A female patient roughly 45 years of age · captured under white-light illumination · the lesion is located on the left thigh · The lesion-visualizer software estimated an average lesion color of about L≈46 a*≈17 b*≈21 (CIELAB), roughly 22 lightness units darker than nearby skin, and a normalized lesion–skin contrast near 14.5. It also reported a border-irregularity rating of about 2/10, internal color variation of about 5.5 on a 0–10 scale, and peripheral color asymmetry of about 1.5 · cropped from a whole-body photographic skin survey; the tile spans about 15 mm.
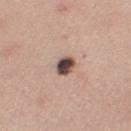{
  "diagnosis": {
    "histopathology": "dysplastic (Clark) nevus",
    "malignancy": "benign",
    "taxonomic_path": [
      "Benign",
      "Benign melanocytic proliferations",
      "Nevus",
      "Nevus, Atypical, Dysplastic, or Clark"
    ]
  }
}